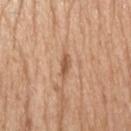Captured during whole-body skin photography for melanoma surveillance; the lesion was not biopsied. The subject is a male aged 43–47. A 15 mm close-up tile from a total-body photography series done for melanoma screening. The lesion is located on the head or neck. Captured under white-light illumination. About 3 mm across.